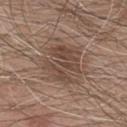Longest diameter approximately 5 mm. A male subject aged approximately 80. The lesion is located on the chest. A 15 mm crop from a total-body photograph taken for skin-cancer surveillance.